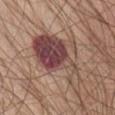Clinical impression: Recorded during total-body skin imaging; not selected for excision or biopsy.